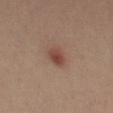The lesion was photographed on a routine skin check and not biopsied; there is no pathology result.